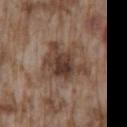Imaged during a routine full-body skin examination; the lesion was not biopsied and no histopathology is available. About 6 mm across. The lesion is located on the lower back. A male patient, aged approximately 75. The tile uses white-light illumination. The lesion-visualizer software estimated a shape-asymmetry score of about 0.4 (0 = symmetric). The analysis additionally found a border-irregularity index near 5.5/10, a color-variation rating of about 6.5/10, and a peripheral color-asymmetry measure near 2. And it measured lesion-presence confidence of about 100/100. Cropped from a total-body skin-imaging series; the visible field is about 15 mm.